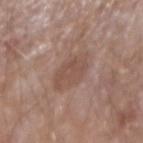{"biopsy_status": "not biopsied; imaged during a skin examination", "site": "arm", "image": {"source": "total-body photography crop", "field_of_view_mm": 15}, "automated_metrics": {"area_mm2_approx": 11.0, "eccentricity": 0.65, "shape_asymmetry": 0.2, "nevus_likeness_0_100": 5, "lesion_detection_confidence_0_100": 100}, "lighting": "white-light", "patient": {"sex": "male", "age_approx": 65}, "lesion_size": {"long_diameter_mm_approx": 4.5}}